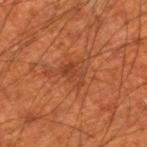Q: Is there a histopathology result?
A: imaged on a skin check; not biopsied
Q: What lighting was used for the tile?
A: cross-polarized
Q: Lesion size?
A: about 3 mm
Q: Patient demographics?
A: male, aged around 70
Q: Lesion location?
A: the right thigh
Q: How was this image acquired?
A: 15 mm crop, total-body photography